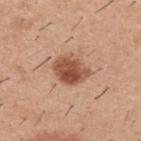<tbp_lesion>
  <biopsy_status>not biopsied; imaged during a skin examination</biopsy_status>
  <image>
    <source>total-body photography crop</source>
    <field_of_view_mm>15</field_of_view_mm>
  </image>
  <patient>
    <sex>male</sex>
    <age_approx>40</age_approx>
  </patient>
  <site>upper back</site>
</tbp_lesion>